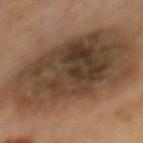{
  "lighting": "cross-polarized",
  "site": "back",
  "image": {
    "source": "total-body photography crop",
    "field_of_view_mm": 15
  },
  "patient": {
    "sex": "male",
    "age_approx": 65
  }
}